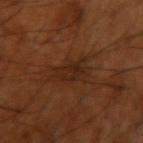Captured during whole-body skin photography for melanoma surveillance; the lesion was not biopsied. From the arm. The lesion-visualizer software estimated an area of roughly 7 mm² and two-axis asymmetry of about 0.35. The software also gave border irregularity of about 5 on a 0–10 scale, a color-variation rating of about 3.5/10, and peripheral color asymmetry of about 1.5. The patient is a male in their 70s. Measured at roughly 4.5 mm in maximum diameter. Cropped from a total-body skin-imaging series; the visible field is about 15 mm.